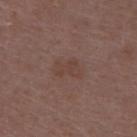  biopsy_status: not biopsied; imaged during a skin examination
  image:
    source: total-body photography crop
    field_of_view_mm: 15
  patient:
    sex: female
    age_approx: 45
  site: back
  lighting: white-light
  lesion_size:
    long_diameter_mm_approx: 3.5